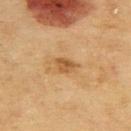– notes: catalogued during a skin exam; not biopsied
– imaging modality: ~15 mm tile from a whole-body skin photo
– patient: male, aged 68–72
– anatomic site: the back